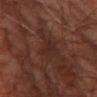Acquisition and patient details: This image is a 15 mm lesion crop taken from a total-body photograph. About 3 mm across. Located on the leg. A male patient roughly 60 years of age.